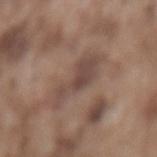Q: Was a biopsy performed?
A: imaged on a skin check; not biopsied
Q: What lighting was used for the tile?
A: white-light
Q: What kind of image is this?
A: total-body-photography crop, ~15 mm field of view
Q: How large is the lesion?
A: ≈5.5 mm
Q: What is the anatomic site?
A: the lower back
Q: What did automated image analysis measure?
A: an area of roughly 11 mm², an outline eccentricity of about 0.9 (0 = round, 1 = elongated), and two-axis asymmetry of about 0.35; an average lesion color of about L≈46 a*≈17 b*≈22 (CIELAB), a lesion–skin lightness drop of about 8, and a lesion-to-skin contrast of about 6.5 (normalized; higher = more distinct); a nevus-likeness score of about 0/100 and lesion-presence confidence of about 85/100
Q: Patient demographics?
A: male, in their mid- to late 70s